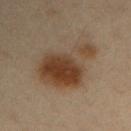The lesion was photographed on a routine skin check and not biopsied; there is no pathology result.
Automated image analysis of the tile measured a border-irregularity index near 5/10, a color-variation rating of about 7.5/10, and radial color variation of about 2.5. The analysis additionally found lesion-presence confidence of about 100/100.
The tile uses cross-polarized illumination.
A 15 mm crop from a total-body photograph taken for skin-cancer surveillance.
Approximately 7 mm at its widest.
A male patient aged 33–37.
On the arm.